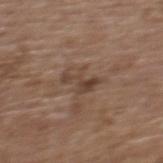biopsy status — catalogued during a skin exam; not biopsied
subject — female, aged around 65
diameter — ≈3.5 mm
body site — the upper back
image source — 15 mm crop, total-body photography
automated lesion analysis — a footprint of about 5 mm², an outline eccentricity of about 0.8 (0 = round, 1 = elongated), and a shape-asymmetry score of about 0.6 (0 = symmetric); an average lesion color of about L≈42 a*≈16 b*≈25 (CIELAB), about 8 CIELAB-L* units darker than the surrounding skin, and a normalized border contrast of about 7; a border-irregularity rating of about 9.5/10 and radial color variation of about 1